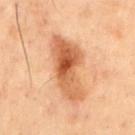Recorded during total-body skin imaging; not selected for excision or biopsy.
This image is a 15 mm lesion crop taken from a total-body photograph.
The lesion is located on the chest.
A male subject aged 53 to 57.